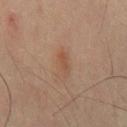<tbp_lesion>
<lesion_size>
  <long_diameter_mm_approx>3.5</long_diameter_mm_approx>
</lesion_size>
<lighting>cross-polarized</lighting>
<patient>
  <sex>male</sex>
  <age_approx>60</age_approx>
</patient>
<image>
  <source>total-body photography crop</source>
  <field_of_view_mm>15</field_of_view_mm>
</image>
<site>mid back</site>
</tbp_lesion>